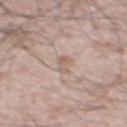Captured during whole-body skin photography for melanoma surveillance; the lesion was not biopsied. The tile uses white-light illumination. Approximately 2.5 mm at its widest. The subject is a male aged approximately 65. The lesion is on the chest. A lesion tile, about 15 mm wide, cut from a 3D total-body photograph.